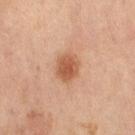Assessment: Imaged during a routine full-body skin examination; the lesion was not biopsied and no histopathology is available. Image and clinical context: Cropped from a whole-body photographic skin survey; the tile spans about 15 mm. A female subject, aged 58 to 62. The lesion is on the right thigh.